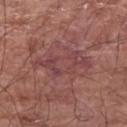| key | value |
|---|---|
| notes | total-body-photography surveillance lesion; no biopsy |
| body site | the left upper arm |
| lighting | white-light illumination |
| lesion diameter | about 7 mm |
| image source | ~15 mm crop, total-body skin-cancer survey |
| patient | male, aged 63–67 |